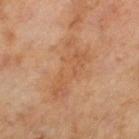Imaged during a routine full-body skin examination; the lesion was not biopsied and no histopathology is available.
The recorded lesion diameter is about 7 mm.
Cropped from a total-body skin-imaging series; the visible field is about 15 mm.
This is a cross-polarized tile.
A male subject aged around 65.
Automated image analysis of the tile measured an average lesion color of about L≈54 a*≈22 b*≈35 (CIELAB), about 7 CIELAB-L* units darker than the surrounding skin, and a lesion-to-skin contrast of about 5 (normalized; higher = more distinct). And it measured a border-irregularity rating of about 7.5/10, a within-lesion color-variation index near 3.5/10, and a peripheral color-asymmetry measure near 1.5. And it measured a lesion-detection confidence of about 100/100.